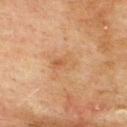Q: Is there a histopathology result?
A: imaged on a skin check; not biopsied
Q: How was this image acquired?
A: ~15 mm crop, total-body skin-cancer survey
Q: Lesion location?
A: the upper back
Q: Who is the patient?
A: male, aged around 75